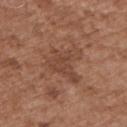Assessment: No biopsy was performed on this lesion — it was imaged during a full skin examination and was not determined to be concerning. Image and clinical context: The tile uses white-light illumination. Approximately 5 mm at its widest. The subject is a male aged 73–77. From the left upper arm. A 15 mm crop from a total-body photograph taken for skin-cancer surveillance.